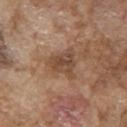notes=catalogued during a skin exam; not biopsied | anatomic site=the right upper arm | image=~15 mm crop, total-body skin-cancer survey | lighting=white-light | size=about 4 mm | subject=male, aged around 75.